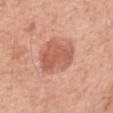<record>
<biopsy_status>not biopsied; imaged during a skin examination</biopsy_status>
</record>